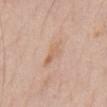Part of a total-body skin-imaging series; this lesion was reviewed on a skin check and was not flagged for biopsy.
The recorded lesion diameter is about 3.5 mm.
Captured under white-light illumination.
A male subject, aged 68–72.
The lesion is located on the chest.
A roughly 15 mm field-of-view crop from a total-body skin photograph.